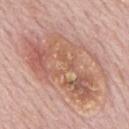notes = no biopsy performed (imaged during a skin exam)
lesion diameter = ~11.5 mm (longest diameter)
patient = male, aged 73–77
image-analysis metrics = two-axis asymmetry of about 0.2; a mean CIELAB color near L≈60 a*≈21 b*≈28, a lesion–skin lightness drop of about 9, and a normalized border contrast of about 6.5; a classifier nevus-likeness of about 10/100
imaging modality = ~15 mm crop, total-body skin-cancer survey
site = the mid back